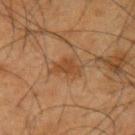Findings:
- workup — imaged on a skin check; not biopsied
- patient — male, in their mid- to late 60s
- illumination — cross-polarized
- body site — the left upper arm
- image-analysis metrics — an average lesion color of about L≈37 a*≈18 b*≈31 (CIELAB), about 7 CIELAB-L* units darker than the surrounding skin, and a normalized border contrast of about 6.5; lesion-presence confidence of about 100/100
- image — total-body-photography crop, ~15 mm field of view